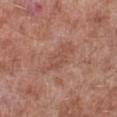notes — imaged on a skin check; not biopsied
illumination — white-light illumination
automated lesion analysis — a lesion area of about 8.5 mm², a shape eccentricity near 0.95, and a shape-asymmetry score of about 0.35 (0 = symmetric); a detector confidence of about 85 out of 100 that the crop contains a lesion
imaging modality — 15 mm crop, total-body photography
site — the leg
subject — male, aged approximately 55